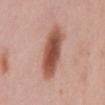This lesion was catalogued during total-body skin photography and was not selected for biopsy. Cropped from a whole-body photographic skin survey; the tile spans about 15 mm. From the mid back. Measured at roughly 7.5 mm in maximum diameter. A female subject aged around 50. The tile uses white-light illumination.